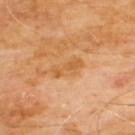Q: Was a biopsy performed?
A: imaged on a skin check; not biopsied
Q: How large is the lesion?
A: about 3.5 mm
Q: Who is the patient?
A: male, aged 58 to 62
Q: What kind of image is this?
A: ~15 mm tile from a whole-body skin photo
Q: Illumination type?
A: cross-polarized illumination
Q: What is the anatomic site?
A: the chest